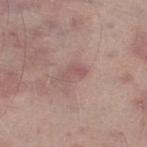The lesion was tiled from a total-body skin photograph and was not biopsied.
A male patient aged approximately 65.
The lesion's longest dimension is about 3 mm.
This is a white-light tile.
Automated tile analysis of the lesion measured an area of roughly 3.5 mm² and two-axis asymmetry of about 0.55. The analysis additionally found a mean CIELAB color near L≈54 a*≈21 b*≈20, a lesion–skin lightness drop of about 7, and a normalized lesion–skin contrast near 5. The software also gave a border-irregularity index near 5/10 and peripheral color asymmetry of about 0. It also reported a detector confidence of about 100 out of 100 that the crop contains a lesion.
On the left lower leg.
A region of skin cropped from a whole-body photographic capture, roughly 15 mm wide.